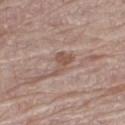{"biopsy_status": "not biopsied; imaged during a skin examination", "automated_metrics": {"nevus_likeness_0_100": 0, "lesion_detection_confidence_0_100": 100}, "patient": {"sex": "male", "age_approx": 65}, "image": {"source": "total-body photography crop", "field_of_view_mm": 15}, "site": "right thigh"}